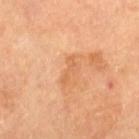* workup · catalogued during a skin exam; not biopsied
* patient · female, about 70 years old
* location · the left thigh
* imaging modality · ~15 mm tile from a whole-body skin photo